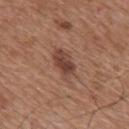Captured during whole-body skin photography for melanoma surveillance; the lesion was not biopsied.